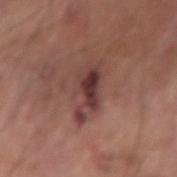biopsy status: no biopsy performed (imaged during a skin exam)